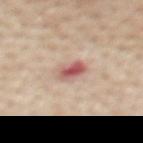Q: Was a biopsy performed?
A: catalogued during a skin exam; not biopsied